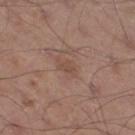Notes:
• follow-up · total-body-photography surveillance lesion; no biopsy
• size · about 3 mm
• body site · the right thigh
• imaging modality · ~15 mm tile from a whole-body skin photo
• illumination · white-light
• subject · male, aged approximately 55